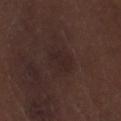{
  "biopsy_status": "not biopsied; imaged during a skin examination",
  "lesion_size": {
    "long_diameter_mm_approx": 3.5
  },
  "lighting": "white-light",
  "image": {
    "source": "total-body photography crop",
    "field_of_view_mm": 15
  },
  "site": "right lower leg",
  "patient": {
    "sex": "male",
    "age_approx": 70
  }
}